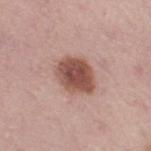Clinical impression:
Imaged during a routine full-body skin examination; the lesion was not biopsied and no histopathology is available.